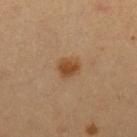A female subject in their mid- to late 30s. This is a cross-polarized tile. The lesion is located on the left forearm. A region of skin cropped from a whole-body photographic capture, roughly 15 mm wide.